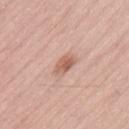workup=catalogued during a skin exam; not biopsied
image=15 mm crop, total-body photography
patient=male, aged around 55
tile lighting=white-light illumination
lesion diameter=≈3 mm
TBP lesion metrics=a lesion area of about 4.5 mm², a shape eccentricity near 0.8, and two-axis asymmetry of about 0.3; an average lesion color of about L≈60 a*≈22 b*≈28 (CIELAB), about 11 CIELAB-L* units darker than the surrounding skin, and a normalized border contrast of about 7; a nevus-likeness score of about 45/100 and lesion-presence confidence of about 100/100
anatomic site=the lower back